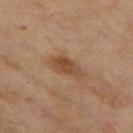The subject is a female aged approximately 60. The recorded lesion diameter is about 4.5 mm. This is a cross-polarized tile. The lesion is on the left thigh. The lesion-visualizer software estimated an average lesion color of about L≈42 a*≈18 b*≈30 (CIELAB), a lesion–skin lightness drop of about 9, and a normalized border contrast of about 7.5. A lesion tile, about 15 mm wide, cut from a 3D total-body photograph.